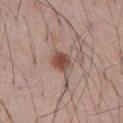Part of a total-body skin-imaging series; this lesion was reviewed on a skin check and was not flagged for biopsy.
A 15 mm crop from a total-body photograph taken for skin-cancer surveillance.
The recorded lesion diameter is about 2.5 mm.
This is a white-light tile.
The lesion is located on the front of the torso.
A male subject, in their mid- to late 50s.